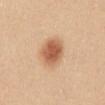biopsy_status: not biopsied; imaged during a skin examination
lesion_size:
  long_diameter_mm_approx: 4.0
image:
  source: total-body photography crop
  field_of_view_mm: 15
patient:
  sex: female
  age_approx: 25
automated_metrics:
  eccentricity: 0.6
  shape_asymmetry: 0.15
  cielab_L: 60
  cielab_a: 23
  cielab_b: 35
  vs_skin_darker_L: 14.0
  vs_skin_contrast_norm: 9.0
  nevus_likeness_0_100: 100
  lesion_detection_confidence_0_100: 100
site: mid back
lighting: white-light